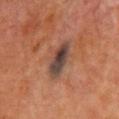Clinical impression: Captured during whole-body skin photography for melanoma surveillance; the lesion was not biopsied. Clinical summary: Located on the chest. A 15 mm close-up tile from a total-body photography series done for melanoma screening. The tile uses cross-polarized illumination. The lesion's longest dimension is about 5.5 mm. The subject is a female aged 78–82.